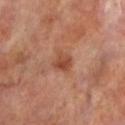Findings:
* follow-up — imaged on a skin check; not biopsied
* lesion diameter — ≈3 mm
* automated lesion analysis — an area of roughly 5 mm², an eccentricity of roughly 0.6, and a symmetry-axis asymmetry near 0.25; a mean CIELAB color near L≈46 a*≈25 b*≈31, roughly 9 lightness units darker than nearby skin, and a normalized lesion–skin contrast near 7; a border-irregularity rating of about 2.5/10
* image — total-body-photography crop, ~15 mm field of view
* location — the left lower leg
* patient — male, approximately 70 years of age